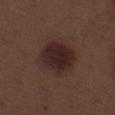workup=imaged on a skin check; not biopsied | patient=male, about 70 years old | TBP lesion metrics=an average lesion color of about L≈24 a*≈17 b*≈18 (CIELAB) and a lesion–skin lightness drop of about 10; a border-irregularity rating of about 1.5/10, a color-variation rating of about 3.5/10, and radial color variation of about 1 | image=~15 mm crop, total-body skin-cancer survey | body site=the left thigh | lesion diameter=≈4.5 mm.